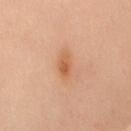Q: Was a biopsy performed?
A: no biopsy performed (imaged during a skin exam)
Q: Who is the patient?
A: female, aged 58–62
Q: What kind of image is this?
A: ~15 mm crop, total-body skin-cancer survey
Q: Where on the body is the lesion?
A: the mid back
Q: What is the lesion's diameter?
A: about 3.5 mm